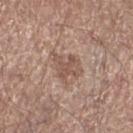The lesion was photographed on a routine skin check and not biopsied; there is no pathology result.
The lesion is on the leg.
A male patient in their 70s.
Cropped from a whole-body photographic skin survey; the tile spans about 15 mm.